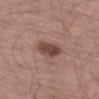Impression:
The lesion was photographed on a routine skin check and not biopsied; there is no pathology result.
Context:
A male patient, aged 63–67. This image is a 15 mm lesion crop taken from a total-body photograph. Longest diameter approximately 3.5 mm. An algorithmic analysis of the crop reported a classifier nevus-likeness of about 80/100 and a detector confidence of about 100 out of 100 that the crop contains a lesion. This is a white-light tile. Located on the left thigh.